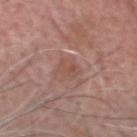Q: Was this lesion biopsied?
A: no biopsy performed (imaged during a skin exam)
Q: What lighting was used for the tile?
A: white-light
Q: What are the patient's age and sex?
A: male, aged 73 to 77
Q: How was this image acquired?
A: 15 mm crop, total-body photography
Q: Lesion location?
A: the head or neck